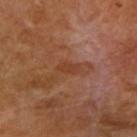Q: Was this lesion biopsied?
A: imaged on a skin check; not biopsied
Q: What is the imaging modality?
A: ~15 mm tile from a whole-body skin photo
Q: How large is the lesion?
A: ~2.5 mm (longest diameter)
Q: Patient demographics?
A: female, about 55 years old
Q: What lighting was used for the tile?
A: cross-polarized illumination
Q: Lesion location?
A: the right upper arm